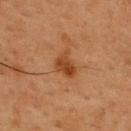Case summary:
- imaging modality: total-body-photography crop, ~15 mm field of view
- size: about 3 mm
- patient: male, roughly 55 years of age
- tile lighting: cross-polarized illumination
- location: the upper back